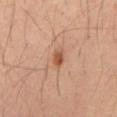The lesion was tiled from a total-body skin photograph and was not biopsied. A male subject aged around 50. The recorded lesion diameter is about 2 mm. A 15 mm close-up extracted from a 3D total-body photography capture. From the mid back.